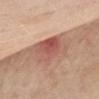workup: catalogued during a skin exam; not biopsied | location: the chest | image: ~15 mm tile from a whole-body skin photo | size: ≈5.5 mm | TBP lesion metrics: a mean CIELAB color near L≈55 a*≈24 b*≈27, a lesion–skin lightness drop of about 12, and a lesion-to-skin contrast of about 8 (normalized; higher = more distinct); a border-irregularity index near 2.5/10 and internal color variation of about 6.5 on a 0–10 scale | patient: female, aged 63 to 67.